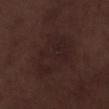Notes:
– image — ~15 mm crop, total-body skin-cancer survey
– diameter — ~7 mm (longest diameter)
– subject — male, about 70 years old
– tile lighting — white-light illumination
– location — the left lower leg